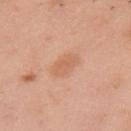Cropped from a total-body skin-imaging series; the visible field is about 15 mm. On the front of the torso. Automated image analysis of the tile measured an area of roughly 6 mm², an outline eccentricity of about 0.8 (0 = round, 1 = elongated), and two-axis asymmetry of about 0.2. The analysis additionally found an average lesion color of about L≈63 a*≈23 b*≈34 (CIELAB), a lesion–skin lightness drop of about 7, and a normalized lesion–skin contrast near 5. The software also gave a border-irregularity rating of about 2/10, internal color variation of about 1.5 on a 0–10 scale, and a peripheral color-asymmetry measure near 0.5. It also reported a nevus-likeness score of about 25/100 and a lesion-detection confidence of about 100/100. A female subject about 50 years old. Measured at roughly 3.5 mm in maximum diameter. This is a white-light tile.